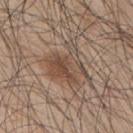Assessment: Recorded during total-body skin imaging; not selected for excision or biopsy. Acquisition and patient details: The lesion-visualizer software estimated a footprint of about 16 mm² and a shape eccentricity near 0.55. It also reported a lesion color around L≈48 a*≈16 b*≈26 in CIELAB, roughly 9 lightness units darker than nearby skin, and a normalized lesion–skin contrast near 7. A male patient in their mid-40s. The recorded lesion diameter is about 5.5 mm. A roughly 15 mm field-of-view crop from a total-body skin photograph. Captured under white-light illumination. On the upper back.